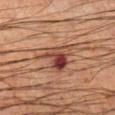Captured during whole-body skin photography for melanoma surveillance; the lesion was not biopsied. A male subject, aged 33 to 37. Cropped from a whole-body photographic skin survey; the tile spans about 15 mm. On the right lower leg. Automated tile analysis of the lesion measured a border-irregularity index near 5.5/10 and radial color variation of about 4. And it measured an automated nevus-likeness rating near 55 out of 100. Captured under cross-polarized illumination.